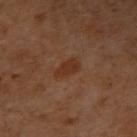Clinical impression:
This lesion was catalogued during total-body skin photography and was not selected for biopsy.